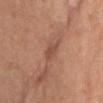Q: Was this lesion biopsied?
A: catalogued during a skin exam; not biopsied
Q: How was this image acquired?
A: 15 mm crop, total-body photography
Q: Lesion size?
A: ≈3.5 mm
Q: What is the anatomic site?
A: the head or neck
Q: What are the patient's age and sex?
A: female, about 75 years old
Q: Illumination type?
A: white-light illumination